The lesion's longest dimension is about 5 mm. This is a white-light tile. Automated image analysis of the tile measured a lesion area of about 10 mm², a shape eccentricity near 0.85, and a shape-asymmetry score of about 0.2 (0 = symmetric). The analysis additionally found a detector confidence of about 100 out of 100 that the crop contains a lesion. The lesion is on the chest. A female subject aged 43–47. A lesion tile, about 15 mm wide, cut from a 3D total-body photograph:
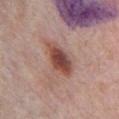Q: What is the histopathologic diagnosis?
A: a dysplastic (Clark) nevus